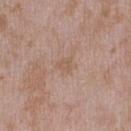Clinical impression:
No biopsy was performed on this lesion — it was imaged during a full skin examination and was not determined to be concerning.
Background:
A 15 mm crop from a total-body photograph taken for skin-cancer surveillance. A male patient, aged approximately 45. This is a white-light tile. Located on the right upper arm.